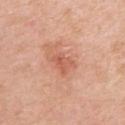Impression: This lesion was catalogued during total-body skin photography and was not selected for biopsy. Clinical summary: The tile uses white-light illumination. The subject is a female approximately 40 years of age. A 15 mm crop from a total-body photograph taken for skin-cancer surveillance. On the right upper arm.